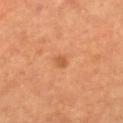follow-up: no biopsy performed (imaged during a skin exam) | diameter: about 2 mm | patient: female, in their 50s | image source: ~15 mm tile from a whole-body skin photo | image-analysis metrics: two-axis asymmetry of about 0.3; an automated nevus-likeness rating near 40 out of 100 and lesion-presence confidence of about 100/100 | body site: the right lower leg.